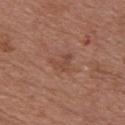This is a white-light tile. Located on the chest. A roughly 15 mm field-of-view crop from a total-body skin photograph. A male subject in their mid-50s.